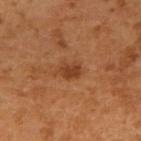Impression:
Imaged during a routine full-body skin examination; the lesion was not biopsied and no histopathology is available.
Context:
A 15 mm close-up tile from a total-body photography series done for melanoma screening. From the right upper arm. A male patient roughly 50 years of age.